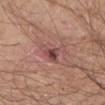This lesion was catalogued during total-body skin photography and was not selected for biopsy. The total-body-photography lesion software estimated an area of roughly 7 mm², an outline eccentricity of about 0.6 (0 = round, 1 = elongated), and two-axis asymmetry of about 0.5. It also reported an average lesion color of about L≈48 a*≈24 b*≈21 (CIELAB), about 9 CIELAB-L* units darker than the surrounding skin, and a normalized lesion–skin contrast near 7.5. A region of skin cropped from a whole-body photographic capture, roughly 15 mm wide. This is a white-light tile. The lesion is on the right forearm. Measured at roughly 3.5 mm in maximum diameter. The subject is a male aged 58–62.